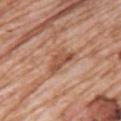The lesion was photographed on a routine skin check and not biopsied; there is no pathology result.
Measured at roughly 4 mm in maximum diameter.
The tile uses white-light illumination.
An algorithmic analysis of the crop reported a footprint of about 6 mm², a shape eccentricity near 0.85, and two-axis asymmetry of about 0.4. It also reported an average lesion color of about L≈52 a*≈23 b*≈32 (CIELAB), a lesion–skin lightness drop of about 11, and a normalized lesion–skin contrast near 7.5. And it measured a color-variation rating of about 2.5/10 and radial color variation of about 1. And it measured lesion-presence confidence of about 100/100.
Cropped from a total-body skin-imaging series; the visible field is about 15 mm.
From the back.
A female subject aged around 70.